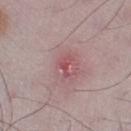| field | value |
|---|---|
| biopsy status | catalogued during a skin exam; not biopsied |
| illumination | white-light |
| lesion size | about 3 mm |
| image source | total-body-photography crop, ~15 mm field of view |
| anatomic site | the left thigh |
| subject | male, aged around 50 |